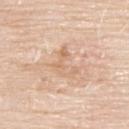Image and clinical context:
The lesion is on the upper back. Automated tile analysis of the lesion measured an area of roughly 7.5 mm² and an eccentricity of roughly 0.7. The analysis additionally found a lesion color around L≈68 a*≈18 b*≈33 in CIELAB, about 7 CIELAB-L* units darker than the surrounding skin, and a normalized lesion–skin contrast near 4.5. The analysis additionally found an automated nevus-likeness rating near 0 out of 100 and a lesion-detection confidence of about 100/100. A male patient approximately 75 years of age. Approximately 4 mm at its widest. Captured under white-light illumination. A close-up tile cropped from a whole-body skin photograph, about 15 mm across.